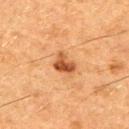| field | value |
|---|---|
| workup | imaged on a skin check; not biopsied |
| location | the right upper arm |
| subject | male, approximately 50 years of age |
| image | ~15 mm tile from a whole-body skin photo |
| diameter | ≈3 mm |